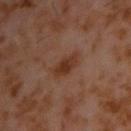The lesion was tiled from a total-body skin photograph and was not biopsied. The lesion is located on the upper back. A male subject in their 60s. A 15 mm close-up extracted from a 3D total-body photography capture. This is a cross-polarized tile.